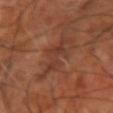Clinical impression: Part of a total-body skin-imaging series; this lesion was reviewed on a skin check and was not flagged for biopsy. Background: A 15 mm crop from a total-body photograph taken for skin-cancer surveillance. The patient is aged 63–67. From the right upper arm.